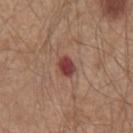workup = catalogued during a skin exam; not biopsied
location = the front of the torso
illumination = white-light
diameter = about 2.5 mm
subject = male, in their mid-60s
image source = 15 mm crop, total-body photography